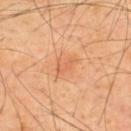This is a cross-polarized tile.
Automated image analysis of the tile measured a shape-asymmetry score of about 0.3 (0 = symmetric). And it measured a border-irregularity index near 3.5/10 and radial color variation of about 0.
The recorded lesion diameter is about 3 mm.
A close-up tile cropped from a whole-body skin photograph, about 15 mm across.
Located on the back.
The patient is a male about 50 years old.